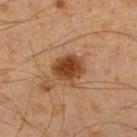| field | value |
|---|---|
| workup | total-body-photography surveillance lesion; no biopsy |
| automated lesion analysis | a footprint of about 9.5 mm², a shape eccentricity near 0.55, and two-axis asymmetry of about 0.15; an average lesion color of about L≈34 a*≈20 b*≈29 (CIELAB) and a lesion-to-skin contrast of about 10.5 (normalized; higher = more distinct); a nevus-likeness score of about 100/100 and a lesion-detection confidence of about 100/100 |
| patient | male, roughly 45 years of age |
| location | the leg |
| image | ~15 mm tile from a whole-body skin photo |